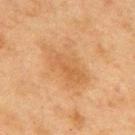{
  "image": {
    "source": "total-body photography crop",
    "field_of_view_mm": 15
  },
  "lighting": "cross-polarized",
  "site": "mid back",
  "patient": {
    "sex": "male",
    "age_approx": 75
  },
  "automated_metrics": {
    "border_irregularity_0_10": 5.5,
    "color_variation_0_10": 2.5,
    "nevus_likeness_0_100": 0,
    "lesion_detection_confidence_0_100": 100
  },
  "lesion_size": {
    "long_diameter_mm_approx": 6.0
  }
}